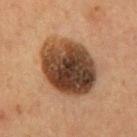The lesion was photographed on a routine skin check and not biopsied; there is no pathology result. The tile uses cross-polarized illumination. A male patient roughly 55 years of age. Measured at roughly 7.5 mm in maximum diameter. Automated tile analysis of the lesion measured an automated nevus-likeness rating near 10 out of 100 and lesion-presence confidence of about 100/100. Located on the mid back. Cropped from a whole-body photographic skin survey; the tile spans about 15 mm.